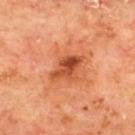This lesion was catalogued during total-body skin photography and was not selected for biopsy. The lesion is located on the upper back. The lesion's longest dimension is about 4 mm. A region of skin cropped from a whole-body photographic capture, roughly 15 mm wide. The patient is a male aged 63–67. The lesion-visualizer software estimated an area of roughly 7 mm² and a symmetry-axis asymmetry near 0.25. It also reported a border-irregularity rating of about 3/10, internal color variation of about 6 on a 0–10 scale, and radial color variation of about 2.5. Imaged with cross-polarized lighting.